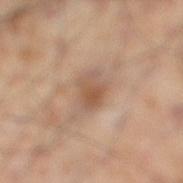Assessment: The lesion was photographed on a routine skin check and not biopsied; there is no pathology result. Background: A male subject, aged approximately 55. The recorded lesion diameter is about 3.5 mm. The lesion-visualizer software estimated an area of roughly 7 mm², an outline eccentricity of about 0.7 (0 = round, 1 = elongated), and a symmetry-axis asymmetry near 0.25. The software also gave a lesion color around L≈55 a*≈18 b*≈30 in CIELAB and about 9 CIELAB-L* units darker than the surrounding skin. And it measured an automated nevus-likeness rating near 30 out of 100. The lesion is located on the right lower leg. This image is a 15 mm lesion crop taken from a total-body photograph.